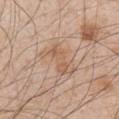The lesion was tiled from a total-body skin photograph and was not biopsied. A close-up tile cropped from a whole-body skin photograph, about 15 mm across. The lesion is located on the right upper arm. An algorithmic analysis of the crop reported an outline eccentricity of about 0.85 (0 = round, 1 = elongated) and a symmetry-axis asymmetry near 0.4. And it measured an average lesion color of about L≈59 a*≈18 b*≈31 (CIELAB) and roughly 7 lightness units darker than nearby skin. It also reported border irregularity of about 6 on a 0–10 scale, a color-variation rating of about 2.5/10, and a peripheral color-asymmetry measure near 0.5. The software also gave an automated nevus-likeness rating near 0 out of 100 and lesion-presence confidence of about 100/100. A male subject, aged 48–52. The lesion's longest dimension is about 5 mm. The tile uses white-light illumination.